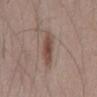The lesion was tiled from a total-body skin photograph and was not biopsied. A male patient, aged around 45. Cropped from a whole-body photographic skin survey; the tile spans about 15 mm. The lesion is on the mid back. This is a white-light tile. Longest diameter approximately 4.5 mm.